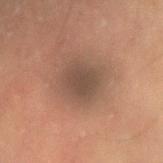A male subject, in their mid-50s.
The tile uses cross-polarized illumination.
A region of skin cropped from a whole-body photographic capture, roughly 15 mm wide.
Longest diameter approximately 3.5 mm.
On the left upper arm.